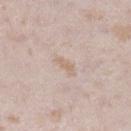No biopsy was performed on this lesion — it was imaged during a full skin examination and was not determined to be concerning. The total-body-photography lesion software estimated a classifier nevus-likeness of about 0/100 and a lesion-detection confidence of about 95/100. A female subject approximately 25 years of age. The lesion's longest dimension is about 3 mm. A 15 mm close-up extracted from a 3D total-body photography capture. The lesion is on the right lower leg. This is a white-light tile.